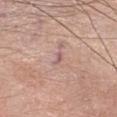biopsy status: imaged on a skin check; not biopsied | body site: the head or neck | size: ~2 mm (longest diameter) | acquisition: ~15 mm tile from a whole-body skin photo | lighting: white-light | subject: female, aged around 60 | TBP lesion metrics: a mean CIELAB color near L≈58 a*≈21 b*≈20 and a lesion-to-skin contrast of about 6.5 (normalized; higher = more distinct); a lesion-detection confidence of about 35/100.